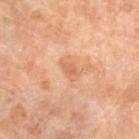This lesion was catalogued during total-body skin photography and was not selected for biopsy.
Measured at roughly 2.5 mm in maximum diameter.
Captured under cross-polarized illumination.
The total-body-photography lesion software estimated a color-variation rating of about 1/10 and peripheral color asymmetry of about 0.5. The analysis additionally found a nevus-likeness score of about 0/100 and lesion-presence confidence of about 100/100.
A female patient, in their 70s.
Located on the left lower leg.
This image is a 15 mm lesion crop taken from a total-body photograph.